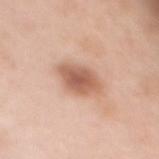This lesion was catalogued during total-body skin photography and was not selected for biopsy.
A lesion tile, about 15 mm wide, cut from a 3D total-body photograph.
A female patient, roughly 50 years of age.
The lesion is on the mid back.
About 5 mm across.
The tile uses white-light illumination.
The total-body-photography lesion software estimated an average lesion color of about L≈60 a*≈22 b*≈31 (CIELAB), roughly 13 lightness units darker than nearby skin, and a normalized border contrast of about 8.5. The analysis additionally found a classifier nevus-likeness of about 75/100.